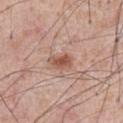The lesion was photographed on a routine skin check and not biopsied; there is no pathology result. The recorded lesion diameter is about 3 mm. Captured under white-light illumination. From the front of the torso. An algorithmic analysis of the crop reported a normalized border contrast of about 8. The software also gave a nevus-likeness score of about 90/100. A 15 mm close-up extracted from a 3D total-body photography capture. The subject is a male about 55 years old.